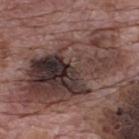Q: Was a biopsy performed?
A: no biopsy performed (imaged during a skin exam)
Q: Automated lesion metrics?
A: a lesion area of about 65 mm², an eccentricity of roughly 0.85, and a symmetry-axis asymmetry near 0.25; a mean CIELAB color near L≈37 a*≈17 b*≈19 and a normalized border contrast of about 10; a within-lesion color-variation index near 10/10 and peripheral color asymmetry of about 5; a lesion-detection confidence of about 80/100
Q: How was the tile lit?
A: white-light
Q: What is the imaging modality?
A: 15 mm crop, total-body photography
Q: Lesion location?
A: the upper back
Q: Patient demographics?
A: male, approximately 70 years of age